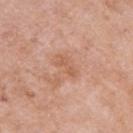Recorded during total-body skin imaging; not selected for excision or biopsy. Longest diameter approximately 2.5 mm. A 15 mm close-up extracted from a 3D total-body photography capture. The total-body-photography lesion software estimated a footprint of about 2.5 mm², an eccentricity of roughly 0.9, and two-axis asymmetry of about 0.35. And it measured an average lesion color of about L≈58 a*≈23 b*≈33 (CIELAB) and about 7 CIELAB-L* units darker than the surrounding skin. The software also gave an automated nevus-likeness rating near 0 out of 100 and lesion-presence confidence of about 100/100. A female subject, roughly 70 years of age. Located on the left upper arm. Imaged with white-light lighting.